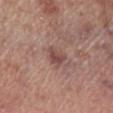| key | value |
|---|---|
| notes | imaged on a skin check; not biopsied |
| patient | male, aged 68 to 72 |
| size | ~2.5 mm (longest diameter) |
| image source | total-body-photography crop, ~15 mm field of view |
| site | the leg |